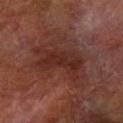Impression: The lesion was photographed on a routine skin check and not biopsied; there is no pathology result. Background: A male subject aged 68 to 72. Located on the right forearm. Cropped from a whole-body photographic skin survey; the tile spans about 15 mm. Approximately 6.5 mm at its widest. Captured under cross-polarized illumination.